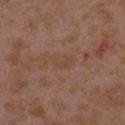Part of a total-body skin-imaging series; this lesion was reviewed on a skin check and was not flagged for biopsy. Cropped from a whole-body photographic skin survey; the tile spans about 15 mm. On the right upper arm. Longest diameter approximately 2.5 mm. A female subject, aged around 35. The total-body-photography lesion software estimated a lesion area of about 2.5 mm² and a symmetry-axis asymmetry near 0.6. It also reported a mean CIELAB color near L≈44 a*≈19 b*≈28, about 4 CIELAB-L* units darker than the surrounding skin, and a lesion-to-skin contrast of about 4.5 (normalized; higher = more distinct).